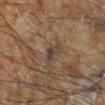workup: catalogued during a skin exam; not biopsied
illumination: cross-polarized
subject: male, approximately 60 years of age
anatomic site: the right lower leg
lesion diameter: ≈3 mm
image: total-body-photography crop, ~15 mm field of view